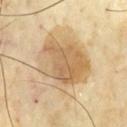{"site": "front of the torso", "image": {"source": "total-body photography crop", "field_of_view_mm": 15}, "lighting": "cross-polarized", "patient": {"sex": "male", "age_approx": 65}, "lesion_size": {"long_diameter_mm_approx": 7.0}}